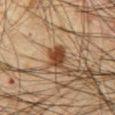Case summary:
– workup · catalogued during a skin exam; not biopsied
– size · ~2.5 mm (longest diameter)
– imaging modality · total-body-photography crop, ~15 mm field of view
– subject · male, aged around 65
– automated lesion analysis · a color-variation rating of about 2.5/10 and radial color variation of about 1; a classifier nevus-likeness of about 95/100 and lesion-presence confidence of about 100/100
– lighting · cross-polarized illumination
– site · the abdomen